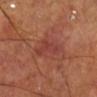Imaged during a routine full-body skin examination; the lesion was not biopsied and no histopathology is available. Automated tile analysis of the lesion measured a color-variation rating of about 2.5/10 and radial color variation of about 1. Cropped from a whole-body photographic skin survey; the tile spans about 15 mm. Measured at roughly 5.5 mm in maximum diameter. This is a cross-polarized tile. A male patient, aged 68 to 72. The lesion is located on the right lower leg.